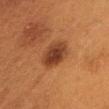Case summary:
- biopsy status: no biopsy performed (imaged during a skin exam)
- body site: the head or neck
- image-analysis metrics: an average lesion color of about L≈33 a*≈20 b*≈29 (CIELAB), a lesion–skin lightness drop of about 10, and a lesion-to-skin contrast of about 9.5 (normalized; higher = more distinct); lesion-presence confidence of about 100/100
- lesion diameter: ≈4 mm
- acquisition: ~15 mm tile from a whole-body skin photo
- patient: female, approximately 40 years of age